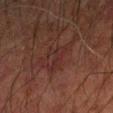Clinical summary: The lesion is located on the right forearm. Imaged with cross-polarized lighting. The subject is a male in their mid-60s. A region of skin cropped from a whole-body photographic capture, roughly 15 mm wide. Approximately 4 mm at its widest. The total-body-photography lesion software estimated a lesion area of about 7.5 mm², an eccentricity of roughly 0.75, and a symmetry-axis asymmetry near 0.55. The analysis additionally found a lesion–skin lightness drop of about 4 and a lesion-to-skin contrast of about 5 (normalized; higher = more distinct). The analysis additionally found a border-irregularity index near 5.5/10 and peripheral color asymmetry of about 1. And it measured a detector confidence of about 80 out of 100 that the crop contains a lesion.